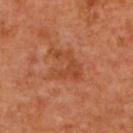Impression: Captured during whole-body skin photography for melanoma surveillance; the lesion was not biopsied. Acquisition and patient details: Cropped from a total-body skin-imaging series; the visible field is about 15 mm. The lesion-visualizer software estimated an eccentricity of roughly 0.65 and a shape-asymmetry score of about 0.6 (0 = symmetric). It also reported a border-irregularity rating of about 8.5/10, a within-lesion color-variation index near 2/10, and peripheral color asymmetry of about 0.5. It also reported an automated nevus-likeness rating near 0 out of 100 and a detector confidence of about 100 out of 100 that the crop contains a lesion. A female subject, roughly 40 years of age. Longest diameter approximately 4 mm. On the upper back. Imaged with cross-polarized lighting.